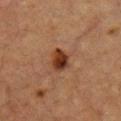Assessment: This lesion was catalogued during total-body skin photography and was not selected for biopsy. Context: The total-body-photography lesion software estimated an area of roughly 6 mm². The analysis additionally found a lesion color around L≈29 a*≈19 b*≈26 in CIELAB, a lesion–skin lightness drop of about 11, and a lesion-to-skin contrast of about 10.5 (normalized; higher = more distinct). The software also gave a color-variation rating of about 5/10. The analysis additionally found a classifier nevus-likeness of about 95/100. A male patient aged around 50. This image is a 15 mm lesion crop taken from a total-body photograph. The lesion's longest dimension is about 3.5 mm. This is a cross-polarized tile. On the chest.